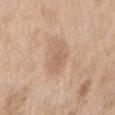Clinical summary:
Automated tile analysis of the lesion measured an area of roughly 9 mm², a shape eccentricity near 0.75, and a symmetry-axis asymmetry near 0.25. The software also gave a lesion color around L≈62 a*≈17 b*≈31 in CIELAB, a lesion–skin lightness drop of about 8, and a lesion-to-skin contrast of about 5 (normalized; higher = more distinct). Longest diameter approximately 4.5 mm. A lesion tile, about 15 mm wide, cut from a 3D total-body photograph. The patient is a female aged 38 to 42. The lesion is on the right upper arm. The tile uses white-light illumination.